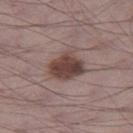Impression:
The lesion was tiled from a total-body skin photograph and was not biopsied.
Context:
A male patient, roughly 70 years of age. Cropped from a total-body skin-imaging series; the visible field is about 15 mm. From the right thigh.